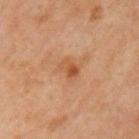{
  "biopsy_status": "not biopsied; imaged during a skin examination",
  "site": "left upper arm",
  "automated_metrics": {
    "cielab_L": 51,
    "cielab_a": 22,
    "cielab_b": 36,
    "vs_skin_darker_L": 8.0,
    "vs_skin_contrast_norm": 6.5,
    "color_variation_0_10": 7.0,
    "peripheral_color_asymmetry": 2.5
  },
  "image": {
    "source": "total-body photography crop",
    "field_of_view_mm": 15
  },
  "patient": {
    "sex": "male",
    "age_approx": 45
  }
}